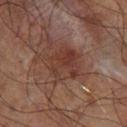Assessment:
Part of a total-body skin-imaging series; this lesion was reviewed on a skin check and was not flagged for biopsy.
Background:
A close-up tile cropped from a whole-body skin photograph, about 15 mm across. A male subject, aged around 70. The lesion-visualizer software estimated an average lesion color of about L≈29 a*≈17 b*≈21 (CIELAB), about 6 CIELAB-L* units darker than the surrounding skin, and a normalized lesion–skin contrast near 6.5. The analysis additionally found border irregularity of about 3.5 on a 0–10 scale and internal color variation of about 5 on a 0–10 scale. From the leg. Imaged with cross-polarized lighting. About 5.5 mm across.